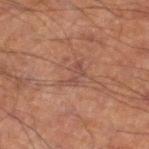lesion size: ~3.5 mm (longest diameter) | subject: male, aged approximately 70 | TBP lesion metrics: a shape eccentricity near 0.85 and a shape-asymmetry score of about 0.6 (0 = symmetric); a mean CIELAB color near L≈39 a*≈18 b*≈22; an automated nevus-likeness rating near 0 out of 100 and lesion-presence confidence of about 95/100 | imaging modality: total-body-photography crop, ~15 mm field of view | body site: the left lower leg.